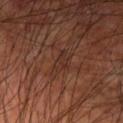The lesion was photographed on a routine skin check and not biopsied; there is no pathology result. A male patient, aged around 60. Located on the left upper arm. The lesion-visualizer software estimated a shape eccentricity near 0.85 and a shape-asymmetry score of about 0.35 (0 = symmetric). It also reported an automated nevus-likeness rating near 0 out of 100 and lesion-presence confidence of about 55/100. Imaged with cross-polarized lighting. A lesion tile, about 15 mm wide, cut from a 3D total-body photograph. The lesion's longest dimension is about 2.5 mm.